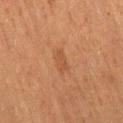follow-up: catalogued during a skin exam; not biopsied | tile lighting: cross-polarized illumination | patient: male, in their mid-70s | acquisition: total-body-photography crop, ~15 mm field of view | body site: the right thigh | automated lesion analysis: a lesion area of about 3 mm², an outline eccentricity of about 0.9 (0 = round, 1 = elongated), and a symmetry-axis asymmetry near 0.25; a border-irregularity index near 2.5/10, a within-lesion color-variation index near 0.5/10, and a peripheral color-asymmetry measure near 0; a classifier nevus-likeness of about 20/100 and a lesion-detection confidence of about 100/100.